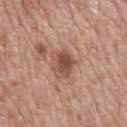Assessment:
Part of a total-body skin-imaging series; this lesion was reviewed on a skin check and was not flagged for biopsy.
Context:
A male subject, approximately 70 years of age. The total-body-photography lesion software estimated an area of roughly 8.5 mm² and a symmetry-axis asymmetry near 0.2. The software also gave a lesion color around L≈51 a*≈21 b*≈27 in CIELAB and a normalized border contrast of about 8. It also reported a border-irregularity index near 2/10, a color-variation rating of about 5.5/10, and radial color variation of about 2. The software also gave a detector confidence of about 100 out of 100 that the crop contains a lesion. On the back. This image is a 15 mm lesion crop taken from a total-body photograph. This is a white-light tile.